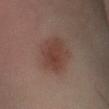| feature | finding |
|---|---|
| biopsy status | catalogued during a skin exam; not biopsied |
| body site | the right lower leg |
| subject | male, roughly 40 years of age |
| imaging modality | total-body-photography crop, ~15 mm field of view |